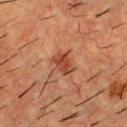The lesion was photographed on a routine skin check and not biopsied; there is no pathology result. A male subject, in their mid- to late 50s. The lesion is located on the upper back. The tile uses cross-polarized illumination. A close-up tile cropped from a whole-body skin photograph, about 15 mm across. The recorded lesion diameter is about 3 mm.